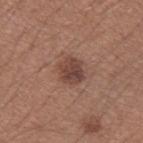Captured during whole-body skin photography for melanoma surveillance; the lesion was not biopsied. Located on the left forearm. A male subject aged 33 to 37. The total-body-photography lesion software estimated a lesion color around L≈43 a*≈20 b*≈24 in CIELAB and roughly 10 lightness units darker than nearby skin. And it measured a border-irregularity index near 2/10, a within-lesion color-variation index near 4/10, and peripheral color asymmetry of about 1.5. A roughly 15 mm field-of-view crop from a total-body skin photograph.